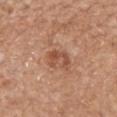Recorded during total-body skin imaging; not selected for excision or biopsy. Measured at roughly 3 mm in maximum diameter. Located on the mid back. The subject is a female aged around 70. A roughly 15 mm field-of-view crop from a total-body skin photograph. This is a white-light tile.